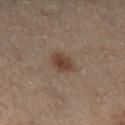The lesion was tiled from a total-body skin photograph and was not biopsied.
The lesion's longest dimension is about 2.5 mm.
Cropped from a whole-body photographic skin survey; the tile spans about 15 mm.
The lesion is on the leg.
The tile uses cross-polarized illumination.
Automated image analysis of the tile measured a shape-asymmetry score of about 0.2 (0 = symmetric). The analysis additionally found a mean CIELAB color near L≈33 a*≈12 b*≈21 and about 7 CIELAB-L* units darker than the surrounding skin. It also reported a border-irregularity index near 1.5/10 and radial color variation of about 0.5. The software also gave a classifier nevus-likeness of about 95/100 and a detector confidence of about 100 out of 100 that the crop contains a lesion.
A male patient, approximately 55 years of age.